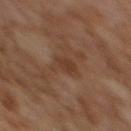| feature | finding |
|---|---|
| notes | imaged on a skin check; not biopsied |
| image | ~15 mm tile from a whole-body skin photo |
| location | the back |
| subject | female, aged approximately 60 |